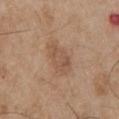Clinical impression:
The lesion was photographed on a routine skin check and not biopsied; there is no pathology result.
Acquisition and patient details:
From the chest. This is a white-light tile. The lesion's longest dimension is about 4 mm. A 15 mm close-up extracted from a 3D total-body photography capture. A male patient aged 53 to 57.